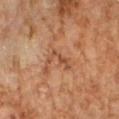No biopsy was performed on this lesion — it was imaged during a full skin examination and was not determined to be concerning. This is a cross-polarized tile. A 15 mm close-up tile from a total-body photography series done for melanoma screening. Automated tile analysis of the lesion measured a footprint of about 3.5 mm², an eccentricity of roughly 0.85, and a symmetry-axis asymmetry near 0.6. It also reported border irregularity of about 8 on a 0–10 scale, a within-lesion color-variation index near 0/10, and a peripheral color-asymmetry measure near 0. A male patient, aged 58 to 62. Measured at roughly 3.5 mm in maximum diameter. Located on the right forearm.